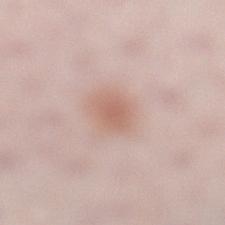No biopsy was performed on this lesion — it was imaged during a full skin examination and was not determined to be concerning.
On the left lower leg.
The subject is a female aged 28 to 32.
Measured at roughly 3 mm in maximum diameter.
Captured under white-light illumination.
A roughly 15 mm field-of-view crop from a total-body skin photograph.